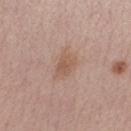Assessment: This lesion was catalogued during total-body skin photography and was not selected for biopsy. Acquisition and patient details: The lesion's longest dimension is about 3 mm. Located on the right forearm. Imaged with white-light lighting. An algorithmic analysis of the crop reported a border-irregularity index near 2/10, a within-lesion color-variation index near 2/10, and a peripheral color-asymmetry measure near 0.5. And it measured a nevus-likeness score of about 5/100 and lesion-presence confidence of about 100/100. A male subject, aged approximately 60. A 15 mm crop from a total-body photograph taken for skin-cancer surveillance.